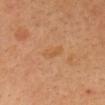Case summary:
• lighting — cross-polarized illumination
• image source — 15 mm crop, total-body photography
• size — ~3 mm (longest diameter)
• location — the head or neck
• patient — female, roughly 40 years of age